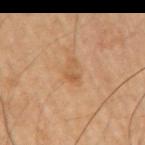Assessment: The lesion was tiled from a total-body skin photograph and was not biopsied. Context: A 15 mm close-up extracted from a 3D total-body photography capture. Automated tile analysis of the lesion measured a footprint of about 3 mm² and an eccentricity of roughly 0.85. The analysis additionally found a lesion color around L≈54 a*≈20 b*≈36 in CIELAB, roughly 7 lightness units darker than nearby skin, and a normalized lesion–skin contrast near 5.5. It also reported border irregularity of about 5.5 on a 0–10 scale, a color-variation rating of about 0/10, and peripheral color asymmetry of about 0. The software also gave a classifier nevus-likeness of about 0/100. Imaged with cross-polarized lighting. Measured at roughly 2.5 mm in maximum diameter. From the right upper arm. The subject is a male about 60 years old.